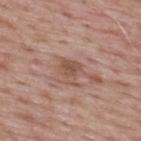Imaged during a routine full-body skin examination; the lesion was not biopsied and no histopathology is available.
Longest diameter approximately 3 mm.
This image is a 15 mm lesion crop taken from a total-body photograph.
A male subject, in their mid-60s.
From the upper back.